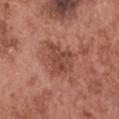{"biopsy_status": "not biopsied; imaged during a skin examination", "site": "head or neck", "image": {"source": "total-body photography crop", "field_of_view_mm": 15}, "automated_metrics": {"eccentricity": 0.25, "shape_asymmetry": 0.4, "cielab_L": 46, "cielab_a": 25, "cielab_b": 28, "vs_skin_darker_L": 9.0, "vs_skin_contrast_norm": 6.5, "peripheral_color_asymmetry": 1.0}, "patient": {"sex": "male", "age_approx": 50}}